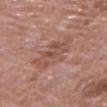Q: Was a biopsy performed?
A: total-body-photography surveillance lesion; no biopsy
Q: What kind of image is this?
A: ~15 mm crop, total-body skin-cancer survey
Q: Patient demographics?
A: female, about 70 years old
Q: Where on the body is the lesion?
A: the arm
Q: What lighting was used for the tile?
A: white-light
Q: Lesion size?
A: ~6 mm (longest diameter)